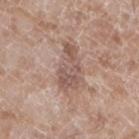image:
  source: total-body photography crop
  field_of_view_mm: 15
patient:
  sex: male
  age_approx: 60
site: leg